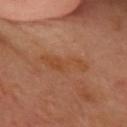biopsy_status: not biopsied; imaged during a skin examination
site: head or neck
patient:
  sex: female
  age_approx: 60
image:
  source: total-body photography crop
  field_of_view_mm: 15